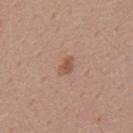{"biopsy_status": "not biopsied; imaged during a skin examination", "patient": {"sex": "male", "age_approx": 55}, "image": {"source": "total-body photography crop", "field_of_view_mm": 15}, "lighting": "white-light", "site": "mid back", "lesion_size": {"long_diameter_mm_approx": 2.5}, "automated_metrics": {"cielab_L": 53, "cielab_a": 21, "cielab_b": 29, "vs_skin_darker_L": 9.0, "vs_skin_contrast_norm": 7.0, "border_irregularity_0_10": 3.0, "color_variation_0_10": 1.5, "peripheral_color_asymmetry": 0.5}}